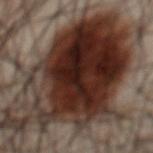Clinical impression: Recorded during total-body skin imaging; not selected for excision or biopsy. Background: The subject is a male approximately 50 years of age. A lesion tile, about 15 mm wide, cut from a 3D total-body photograph. Located on the chest.